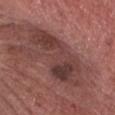Notes:
• follow-up — catalogued during a skin exam; not biopsied
• patient — male, in their 70s
• anatomic site — the head or neck
• tile lighting — white-light illumination
• image source — 15 mm crop, total-body photography